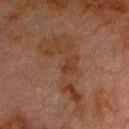Part of a total-body skin-imaging series; this lesion was reviewed on a skin check and was not flagged for biopsy.